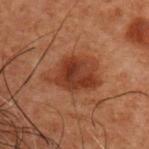On the upper back. Measured at roughly 6 mm in maximum diameter. This image is a 15 mm lesion crop taken from a total-body photograph. A male patient approximately 50 years of age.